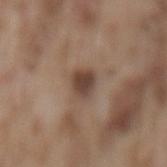Notes:
• workup: no biopsy performed (imaged during a skin exam)
• patient: male, approximately 75 years of age
• TBP lesion metrics: a lesion–skin lightness drop of about 12 and a normalized lesion–skin contrast near 9
• tile lighting: white-light illumination
• lesion size: ≈3 mm
• imaging modality: ~15 mm tile from a whole-body skin photo
• site: the lower back